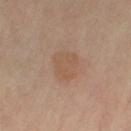Clinical impression: The lesion was tiled from a total-body skin photograph and was not biopsied. Context: A 15 mm close-up tile from a total-body photography series done for melanoma screening. Located on the left thigh. The recorded lesion diameter is about 4 mm. A female subject roughly 50 years of age. This is a cross-polarized tile.